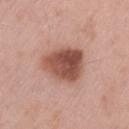Imaged during a routine full-body skin examination; the lesion was not biopsied and no histopathology is available. A roughly 15 mm field-of-view crop from a total-body skin photograph. On the lower back. A male patient, aged approximately 55.